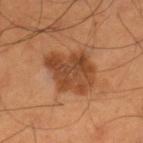| field | value |
|---|---|
| follow-up | imaged on a skin check; not biopsied |
| image | 15 mm crop, total-body photography |
| patient | male, aged 53 to 57 |
| anatomic site | the left thigh |